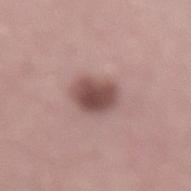  biopsy_status: not biopsied; imaged during a skin examination
  lesion_size:
    long_diameter_mm_approx: 3.5
  site: back
  automated_metrics:
    area_mm2_approx: 9.0
    eccentricity: 0.55
    shape_asymmetry: 0.15
    cielab_L: 48
    cielab_a: 20
    cielab_b: 20
    vs_skin_contrast_norm: 10.0
    border_irregularity_0_10: 1.5
    peripheral_color_asymmetry: 1.0
    nevus_likeness_0_100: 80
    lesion_detection_confidence_0_100: 100
  patient:
    sex: male
    age_approx: 50
  image:
    source: total-body photography crop
    field_of_view_mm: 15